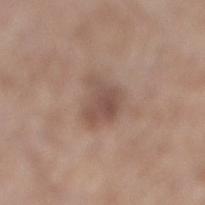Assessment: The lesion was tiled from a total-body skin photograph and was not biopsied. Context: The subject is a male aged around 65. Automated image analysis of the tile measured lesion-presence confidence of about 100/100. This is a white-light tile. A 15 mm close-up extracted from a 3D total-body photography capture. Located on the right lower leg. Measured at roughly 4 mm in maximum diameter.